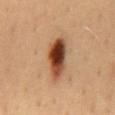Q: Was this lesion biopsied?
A: catalogued during a skin exam; not biopsied
Q: What did automated image analysis measure?
A: a footprint of about 13 mm², a shape eccentricity near 0.9, and a symmetry-axis asymmetry near 0.25; border irregularity of about 2.5 on a 0–10 scale, a color-variation rating of about 10/10, and peripheral color asymmetry of about 3.5; a classifier nevus-likeness of about 100/100
Q: What is the imaging modality?
A: total-body-photography crop, ~15 mm field of view
Q: Who is the patient?
A: male, aged 38 to 42
Q: What is the anatomic site?
A: the lower back